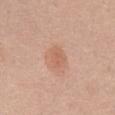subject: female, aged around 60 | diameter: ~3 mm (longest diameter) | location: the abdomen | acquisition: ~15 mm tile from a whole-body skin photo | lighting: white-light illumination.